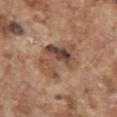biopsy_status: not biopsied; imaged during a skin examination
site: right upper arm
image:
  source: total-body photography crop
  field_of_view_mm: 15
patient:
  sex: male
  age_approx: 75